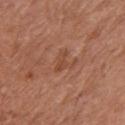workup = no biopsy performed (imaged during a skin exam)
subject = female, aged approximately 65
tile lighting = white-light
location = the arm
image-analysis metrics = border irregularity of about 5 on a 0–10 scale and peripheral color asymmetry of about 0
image = ~15 mm crop, total-body skin-cancer survey